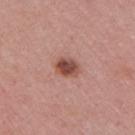Clinical impression:
This lesion was catalogued during total-body skin photography and was not selected for biopsy.
Acquisition and patient details:
A 15 mm close-up tile from a total-body photography series done for melanoma screening. This is a white-light tile. About 2.5 mm across. A female patient aged around 55. The lesion is on the right upper arm. Automated image analysis of the tile measured an area of roughly 5.5 mm² and a symmetry-axis asymmetry near 0.15. The analysis additionally found about 14 CIELAB-L* units darker than the surrounding skin and a normalized border contrast of about 9.5. And it measured lesion-presence confidence of about 100/100.